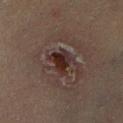tile lighting: cross-polarized illumination | subject: male, about 60 years old | body site: the right lower leg | automated metrics: an area of roughly 6 mm², a shape eccentricity near 0.65, and a shape-asymmetry score of about 0.45 (0 = symmetric); a lesion color around L≈23 a*≈16 b*≈17 in CIELAB and a lesion-to-skin contrast of about 11 (normalized; higher = more distinct); a nevus-likeness score of about 45/100 | lesion diameter: about 3.5 mm | imaging modality: 15 mm crop, total-body photography.